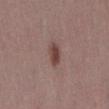The lesion was tiled from a total-body skin photograph and was not biopsied. The tile uses white-light illumination. The lesion is on the right thigh. The subject is a female about 45 years old. This image is a 15 mm lesion crop taken from a total-body photograph. The lesion's longest dimension is about 3 mm.